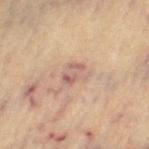Q: Was this lesion biopsied?
A: catalogued during a skin exam; not biopsied
Q: What kind of image is this?
A: 15 mm crop, total-body photography
Q: What are the patient's age and sex?
A: female, aged 63–67
Q: What is the anatomic site?
A: the left thigh